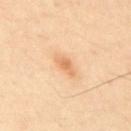Clinical impression: Recorded during total-body skin imaging; not selected for excision or biopsy. Clinical summary: A male subject aged around 50. Automated image analysis of the tile measured border irregularity of about 2.5 on a 0–10 scale, a color-variation rating of about 1.5/10, and a peripheral color-asymmetry measure near 0.5. The software also gave a detector confidence of about 100 out of 100 that the crop contains a lesion. A 15 mm close-up tile from a total-body photography series done for melanoma screening. From the upper back.